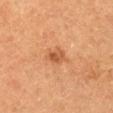Notes:
– notes — imaged on a skin check; not biopsied
– acquisition — 15 mm crop, total-body photography
– patient — female, roughly 50 years of age